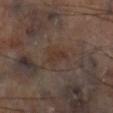Assessment:
The lesion was tiled from a total-body skin photograph and was not biopsied.
Acquisition and patient details:
Approximately 3 mm at its widest. Cropped from a whole-body photographic skin survey; the tile spans about 15 mm. An algorithmic analysis of the crop reported internal color variation of about 2 on a 0–10 scale and peripheral color asymmetry of about 0.5. Captured under cross-polarized illumination. The lesion is located on the right lower leg.